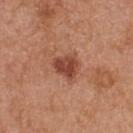Longest diameter approximately 3 mm. Captured under white-light illumination. From the upper back. The patient is a female in their 40s. Cropped from a total-body skin-imaging series; the visible field is about 15 mm. The total-body-photography lesion software estimated a lesion area of about 6.5 mm², a shape eccentricity near 0.45, and two-axis asymmetry of about 0.3. It also reported a border-irregularity index near 3/10, internal color variation of about 3 on a 0–10 scale, and peripheral color asymmetry of about 1. And it measured lesion-presence confidence of about 100/100.